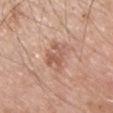- workup — total-body-photography surveillance lesion; no biopsy
- body site — the chest
- acquisition — 15 mm crop, total-body photography
- subject — male, in their mid- to late 50s
- lighting — white-light illumination